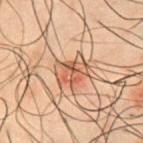notes = imaged on a skin check; not biopsied
subject = male, roughly 50 years of age
lighting = cross-polarized
body site = the chest
lesion diameter = ≈4 mm
acquisition = ~15 mm tile from a whole-body skin photo